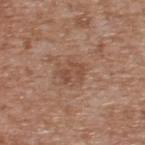Captured during whole-body skin photography for melanoma surveillance; the lesion was not biopsied.